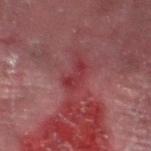No biopsy was performed on this lesion — it was imaged during a full skin examination and was not determined to be concerning. A male patient aged 53 to 57. The lesion is on the left thigh. A close-up tile cropped from a whole-body skin photograph, about 15 mm across.